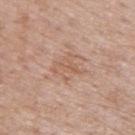Q: Was this lesion biopsied?
A: catalogued during a skin exam; not biopsied
Q: How was this image acquired?
A: ~15 mm crop, total-body skin-cancer survey
Q: How large is the lesion?
A: about 4 mm
Q: Illumination type?
A: white-light
Q: What is the anatomic site?
A: the upper back
Q: What are the patient's age and sex?
A: male, in their 70s